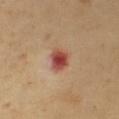The lesion was photographed on a routine skin check and not biopsied; there is no pathology result.
The patient is a male in their mid- to late 60s.
Automated image analysis of the tile measured an area of roughly 5 mm² and an eccentricity of roughly 0.55. And it measured a border-irregularity index near 1.5/10, a color-variation rating of about 5/10, and peripheral color asymmetry of about 1.5.
Captured under cross-polarized illumination.
Longest diameter approximately 2.5 mm.
Cropped from a whole-body photographic skin survey; the tile spans about 15 mm.